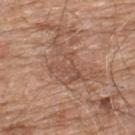Captured during whole-body skin photography for melanoma surveillance; the lesion was not biopsied. About 6.5 mm across. Cropped from a whole-body photographic skin survey; the tile spans about 15 mm. From the upper back. Imaged with white-light lighting. The patient is a male aged approximately 80.